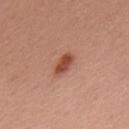No biopsy was performed on this lesion — it was imaged during a full skin examination and was not determined to be concerning.
A male subject aged around 55.
A region of skin cropped from a whole-body photographic capture, roughly 15 mm wide.
The recorded lesion diameter is about 3 mm.
An algorithmic analysis of the crop reported a footprint of about 4 mm² and a symmetry-axis asymmetry near 0.2. It also reported a lesion–skin lightness drop of about 13 and a normalized border contrast of about 9. It also reported a border-irregularity rating of about 2/10, a color-variation rating of about 2.5/10, and peripheral color asymmetry of about 1. It also reported an automated nevus-likeness rating near 100 out of 100 and a detector confidence of about 100 out of 100 that the crop contains a lesion.
The lesion is on the mid back.
Imaged with white-light lighting.